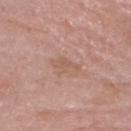| key | value |
|---|---|
| follow-up | imaged on a skin check; not biopsied |
| patient | male, aged 58 to 62 |
| anatomic site | the head or neck |
| imaging modality | 15 mm crop, total-body photography |